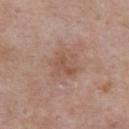Assessment: No biopsy was performed on this lesion — it was imaged during a full skin examination and was not determined to be concerning. Background: On the front of the torso. This is a white-light tile. A 15 mm crop from a total-body photograph taken for skin-cancer surveillance. Longest diameter approximately 4 mm. The total-body-photography lesion software estimated a footprint of about 10 mm² and a symmetry-axis asymmetry near 0.2. It also reported a border-irregularity rating of about 2.5/10 and a peripheral color-asymmetry measure near 1. A male patient aged approximately 55.